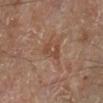workup: imaged on a skin check; not biopsied | image source: 15 mm crop, total-body photography | patient: male, approximately 70 years of age | illumination: cross-polarized illumination | automated metrics: a lesion area of about 3.5 mm², an eccentricity of roughly 0.85, and a shape-asymmetry score of about 0.45 (0 = symmetric); a lesion color around L≈44 a*≈20 b*≈28 in CIELAB, a lesion–skin lightness drop of about 7, and a lesion-to-skin contrast of about 6 (normalized; higher = more distinct); a border-irregularity rating of about 6/10 and internal color variation of about 0 on a 0–10 scale; a nevus-likeness score of about 0/100 and a lesion-detection confidence of about 100/100 | site: the left lower leg | lesion size: ~3 mm (longest diameter).